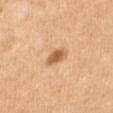Assessment:
Imaged during a routine full-body skin examination; the lesion was not biopsied and no histopathology is available.